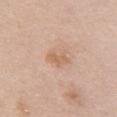Assessment: Captured during whole-body skin photography for melanoma surveillance; the lesion was not biopsied. Background: Located on the chest. Automated tile analysis of the lesion measured an average lesion color of about L≈62 a*≈20 b*≈32 (CIELAB), about 8 CIELAB-L* units darker than the surrounding skin, and a normalized lesion–skin contrast near 6. And it measured internal color variation of about 0 on a 0–10 scale. The subject is a female aged approximately 60. The lesion's longest dimension is about 3 mm. A roughly 15 mm field-of-view crop from a total-body skin photograph.